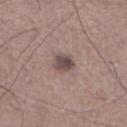The lesion is on the leg.
Automated tile analysis of the lesion measured a lesion area of about 5 mm². And it measured a mean CIELAB color near L≈47 a*≈15 b*≈18, a lesion–skin lightness drop of about 12, and a normalized border contrast of about 9.
A 15 mm close-up tile from a total-body photography series done for melanoma screening.
The subject is a male aged around 70.
This is a white-light tile.
The recorded lesion diameter is about 2.5 mm.